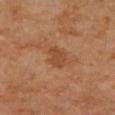Assessment: The lesion was photographed on a routine skin check and not biopsied; there is no pathology result.